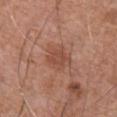notes = total-body-photography surveillance lesion; no biopsy
illumination = white-light illumination
subject = male, aged around 75
lesion diameter = ~3.5 mm (longest diameter)
location = the front of the torso
image source = total-body-photography crop, ~15 mm field of view